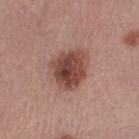Recorded during total-body skin imaging; not selected for excision or biopsy. A female subject, about 50 years old. An algorithmic analysis of the crop reported a color-variation rating of about 6.5/10 and peripheral color asymmetry of about 2.5. The software also gave an automated nevus-likeness rating near 80 out of 100 and a detector confidence of about 100 out of 100 that the crop contains a lesion. The tile uses white-light illumination. On the right thigh. A 15 mm close-up tile from a total-body photography series done for melanoma screening. About 5 mm across.